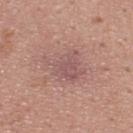No biopsy was performed on this lesion — it was imaged during a full skin examination and was not determined to be concerning. A 15 mm crop from a total-body photograph taken for skin-cancer surveillance. A male subject, about 25 years old. Located on the upper back.